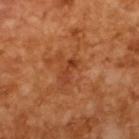- image · ~15 mm crop, total-body skin-cancer survey
- patient · male, aged 63–67